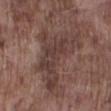{
  "biopsy_status": "not biopsied; imaged during a skin examination",
  "image": {
    "source": "total-body photography crop",
    "field_of_view_mm": 15
  },
  "patient": {
    "sex": "male",
    "age_approx": 75
  },
  "site": "right lower leg"
}